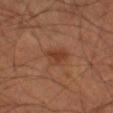Case summary:
* workup · total-body-photography surveillance lesion; no biopsy
* lesion size · ~3 mm (longest diameter)
* patient · male, aged 68–72
* body site · the leg
* tile lighting · cross-polarized illumination
* imaging modality · total-body-photography crop, ~15 mm field of view